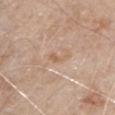Findings:
* biopsy status: total-body-photography surveillance lesion; no biopsy
* size: ~2.5 mm (longest diameter)
* TBP lesion metrics: two-axis asymmetry of about 0.5; a lesion color around L≈61 a*≈18 b*≈32 in CIELAB and about 6 CIELAB-L* units darker than the surrounding skin
* tile lighting: white-light illumination
* image: ~15 mm crop, total-body skin-cancer survey
* site: the front of the torso
* subject: male, about 80 years old